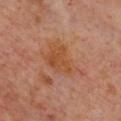This lesion was catalogued during total-body skin photography and was not selected for biopsy. The lesion is on the chest. A 15 mm close-up tile from a total-body photography series done for melanoma screening. The tile uses cross-polarized illumination. A female subject roughly 60 years of age.